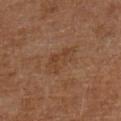Imaged during a routine full-body skin examination; the lesion was not biopsied and no histopathology is available. This image is a 15 mm lesion crop taken from a total-body photograph. The lesion is on the left lower leg. Imaged with cross-polarized lighting. The subject is a male in their 60s. Approximately 4 mm at its widest.